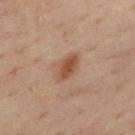Captured during whole-body skin photography for melanoma surveillance; the lesion was not biopsied. The subject is a male aged around 50. The lesion is located on the mid back. A close-up tile cropped from a whole-body skin photograph, about 15 mm across. Imaged with cross-polarized lighting. An algorithmic analysis of the crop reported a detector confidence of about 100 out of 100 that the crop contains a lesion. About 3.5 mm across.